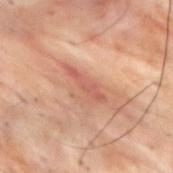- biopsy status — imaged on a skin check; not biopsied
- location — the upper back
- lesion size — ≈5 mm
- patient — male, aged around 30
- automated metrics — border irregularity of about 6 on a 0–10 scale, internal color variation of about 1.5 on a 0–10 scale, and radial color variation of about 0.5; a nevus-likeness score of about 0/100 and a lesion-detection confidence of about 55/100
- imaging modality — ~15 mm crop, total-body skin-cancer survey